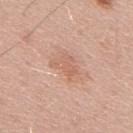Acquisition and patient details:
Captured under white-light illumination. A lesion tile, about 15 mm wide, cut from a 3D total-body photograph. A male patient, approximately 55 years of age. On the mid back. Approximately 3.5 mm at its widest.